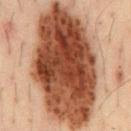notes: catalogued during a skin exam; not biopsied | subject: male, aged around 35 | acquisition: ~15 mm tile from a whole-body skin photo | site: the chest.